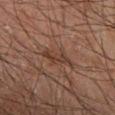Impression:
Captured during whole-body skin photography for melanoma surveillance; the lesion was not biopsied.
Image and clinical context:
On the right forearm. About 4.5 mm across. The subject is a male in their mid- to late 40s. Captured under cross-polarized illumination. A 15 mm close-up tile from a total-body photography series done for melanoma screening.